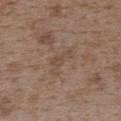Impression:
Imaged during a routine full-body skin examination; the lesion was not biopsied and no histopathology is available.
Clinical summary:
Captured under white-light illumination. Automated tile analysis of the lesion measured a lesion area of about 5 mm² and an eccentricity of roughly 0.9. The software also gave border irregularity of about 7 on a 0–10 scale, internal color variation of about 1 on a 0–10 scale, and radial color variation of about 0.5. And it measured a classifier nevus-likeness of about 0/100. A female patient in their mid-30s. Cropped from a whole-body photographic skin survey; the tile spans about 15 mm. Longest diameter approximately 4 mm. The lesion is on the upper back.